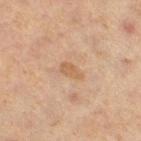Case summary:
• notes · catalogued during a skin exam; not biopsied
• lesion diameter · ~2.5 mm (longest diameter)
• subject · female, aged approximately 60
• site · the right thigh
• image source · total-body-photography crop, ~15 mm field of view
• automated lesion analysis · a detector confidence of about 100 out of 100 that the crop contains a lesion